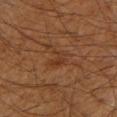Imaged during a routine full-body skin examination; the lesion was not biopsied and no histopathology is available.
Imaged with cross-polarized lighting.
A male patient, in their 60s.
A lesion tile, about 15 mm wide, cut from a 3D total-body photograph.
On the left upper arm.